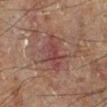This lesion was catalogued during total-body skin photography and was not selected for biopsy.
The subject is a male about 60 years old.
A 15 mm crop from a total-body photograph taken for skin-cancer surveillance.
Located on the left leg.